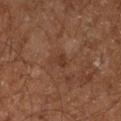Assessment:
Recorded during total-body skin imaging; not selected for excision or biopsy.
Acquisition and patient details:
Imaged with cross-polarized lighting. A roughly 15 mm field-of-view crop from a total-body skin photograph. The lesion is on the left lower leg. A male subject, about 60 years old. The lesion-visualizer software estimated a footprint of about 3 mm² and an eccentricity of roughly 0.8. The software also gave an average lesion color of about L≈32 a*≈18 b*≈26 (CIELAB), about 6 CIELAB-L* units darker than the surrounding skin, and a normalized border contrast of about 5.5. The software also gave border irregularity of about 3.5 on a 0–10 scale, internal color variation of about 1 on a 0–10 scale, and peripheral color asymmetry of about 0.5. The recorded lesion diameter is about 2.5 mm.